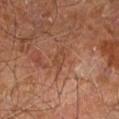{
  "biopsy_status": "not biopsied; imaged during a skin examination",
  "image": {
    "source": "total-body photography crop",
    "field_of_view_mm": 15
  },
  "lesion_size": {
    "long_diameter_mm_approx": 2.5
  },
  "patient": {
    "sex": "male",
    "age_approx": 60
  },
  "automated_metrics": {
    "area_mm2_approx": 3.0,
    "shape_asymmetry": 0.45,
    "cielab_L": 43,
    "cielab_a": 22,
    "cielab_b": 30,
    "vs_skin_darker_L": 5.0,
    "vs_skin_contrast_norm": 4.5,
    "border_irregularity_0_10": 4.0,
    "color_variation_0_10": 2.5,
    "peripheral_color_asymmetry": 1.0
  },
  "site": "right leg",
  "lighting": "cross-polarized"
}